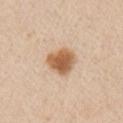• biopsy status: total-body-photography surveillance lesion; no biopsy
• imaging modality: 15 mm crop, total-body photography
• subject: female, aged approximately 50
• location: the right upper arm